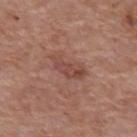Q: Is there a histopathology result?
A: total-body-photography surveillance lesion; no biopsy
Q: What is the anatomic site?
A: the upper back
Q: What is the lesion's diameter?
A: ≈4.5 mm
Q: What is the imaging modality?
A: total-body-photography crop, ~15 mm field of view
Q: What lighting was used for the tile?
A: white-light illumination
Q: Patient demographics?
A: female, aged around 65